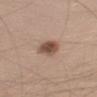notes=catalogued during a skin exam; not biopsied
tile lighting=white-light illumination
subject=male, roughly 30 years of age
lesion size=about 4 mm
imaging modality=~15 mm tile from a whole-body skin photo
body site=the left upper arm